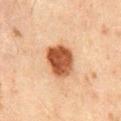<tbp_lesion>
<biopsy_status>not biopsied; imaged during a skin examination</biopsy_status>
<site>mid back</site>
<patient>
  <sex>male</sex>
  <age_approx>45</age_approx>
</patient>
<lesion_size>
  <long_diameter_mm_approx>4.0</long_diameter_mm_approx>
</lesion_size>
<image>
  <source>total-body photography crop</source>
  <field_of_view_mm>15</field_of_view_mm>
</image>
<lighting>cross-polarized</lighting>
</tbp_lesion>